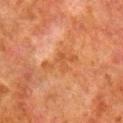Q: Was a biopsy performed?
A: no biopsy performed (imaged during a skin exam)
Q: Automated lesion metrics?
A: an area of roughly 7 mm² and two-axis asymmetry of about 0.45; a normalized lesion–skin contrast near 5.5; border irregularity of about 7 on a 0–10 scale, internal color variation of about 2 on a 0–10 scale, and peripheral color asymmetry of about 0.5; a nevus-likeness score of about 0/100 and lesion-presence confidence of about 95/100
Q: How was the tile lit?
A: cross-polarized
Q: How was this image acquired?
A: 15 mm crop, total-body photography
Q: Who is the patient?
A: male, aged 78 to 82
Q: Where on the body is the lesion?
A: the right lower leg
Q: What is the lesion's diameter?
A: ~5 mm (longest diameter)